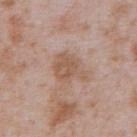Notes:
– follow-up · total-body-photography surveillance lesion; no biopsy
– automated metrics · a border-irregularity index near 4/10, internal color variation of about 2.5 on a 0–10 scale, and radial color variation of about 1
– lesion size · about 4.5 mm
– image source · ~15 mm tile from a whole-body skin photo
– patient · male, about 65 years old
– site · the front of the torso
– tile lighting · white-light illumination